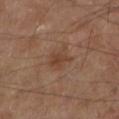The lesion was photographed on a routine skin check and not biopsied; there is no pathology result. This is a cross-polarized tile. The recorded lesion diameter is about 3.5 mm. The subject is a male aged 68–72. The lesion-visualizer software estimated a mean CIELAB color near L≈40 a*≈19 b*≈28 and a lesion–skin lightness drop of about 6. It also reported a within-lesion color-variation index near 3/10. A 15 mm close-up extracted from a 3D total-body photography capture. On the left lower leg.